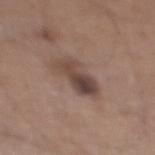image=15 mm crop, total-body photography; patient=male, aged approximately 65; anatomic site=the right lower leg.